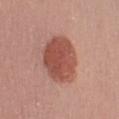The lesion was tiled from a total-body skin photograph and was not biopsied.
A 15 mm close-up tile from a total-body photography series done for melanoma screening.
The patient is a male aged approximately 45.
The lesion is on the left upper arm.
Imaged with white-light lighting.